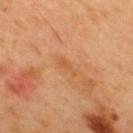– subject: male, aged 48–52
– body site: the upper back
– tile lighting: cross-polarized
– lesion size: ~3 mm (longest diameter)
– acquisition: total-body-photography crop, ~15 mm field of view
– automated lesion analysis: an average lesion color of about L≈57 a*≈25 b*≈42 (CIELAB), roughly 6 lightness units darker than nearby skin, and a lesion-to-skin contrast of about 5 (normalized; higher = more distinct); a border-irregularity index near 3.5/10, internal color variation of about 0 on a 0–10 scale, and peripheral color asymmetry of about 0; a classifier nevus-likeness of about 0/100 and a lesion-detection confidence of about 100/100